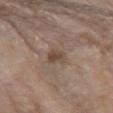Captured during whole-body skin photography for melanoma surveillance; the lesion was not biopsied.